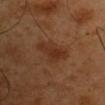The lesion was photographed on a routine skin check and not biopsied; there is no pathology result. On the arm. Imaged with cross-polarized lighting. A 15 mm close-up tile from a total-body photography series done for melanoma screening. Longest diameter approximately 4 mm. A male patient approximately 50 years of age.